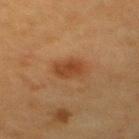Notes:
– biopsy status — imaged on a skin check; not biopsied
– location — the back
– patient — female, aged around 55
– imaging modality — total-body-photography crop, ~15 mm field of view